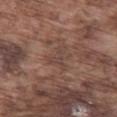patient: male, about 75 years old | image source: ~15 mm crop, total-body skin-cancer survey | size: ~3.5 mm (longest diameter) | tile lighting: white-light | automated metrics: border irregularity of about 8.5 on a 0–10 scale, a color-variation rating of about 0/10, and peripheral color asymmetry of about 0; an automated nevus-likeness rating near 0 out of 100 and lesion-presence confidence of about 60/100 | site: the left thigh.